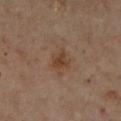Case summary:
– notes: catalogued during a skin exam; not biopsied
– patient: female, aged 58 to 62
– site: the right lower leg
– lighting: cross-polarized
– image source: 15 mm crop, total-body photography
– TBP lesion metrics: a nevus-likeness score of about 45/100 and a lesion-detection confidence of about 100/100
– diameter: about 3 mm